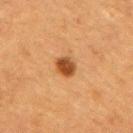This lesion was catalogued during total-body skin photography and was not selected for biopsy. Automated image analysis of the tile measured border irregularity of about 1 on a 0–10 scale, a color-variation rating of about 3/10, and radial color variation of about 1. A region of skin cropped from a whole-body photographic capture, roughly 15 mm wide. The subject is a female aged approximately 55. Longest diameter approximately 2.5 mm. Captured under cross-polarized illumination. The lesion is on the back.